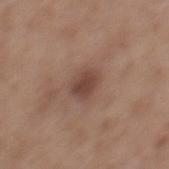  biopsy_status: not biopsied; imaged during a skin examination
  lesion_size:
    long_diameter_mm_approx: 3.0
  patient:
    sex: male
    age_approx: 55
  image:
    source: total-body photography crop
    field_of_view_mm: 15
  site: mid back